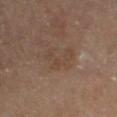Imaged during a routine full-body skin examination; the lesion was not biopsied and no histopathology is available.
A female subject, in their mid- to late 60s.
A close-up tile cropped from a whole-body skin photograph, about 15 mm across.
From the right upper arm.